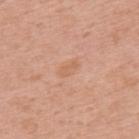Impression: Captured during whole-body skin photography for melanoma surveillance; the lesion was not biopsied. Acquisition and patient details: A male subject roughly 60 years of age. The lesion is on the upper back. This image is a 15 mm lesion crop taken from a total-body photograph.